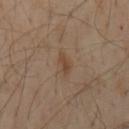Q: Was this lesion biopsied?
A: total-body-photography surveillance lesion; no biopsy
Q: Lesion size?
A: about 3 mm
Q: What did automated image analysis measure?
A: a lesion color around L≈44 a*≈16 b*≈29 in CIELAB, a lesion–skin lightness drop of about 7, and a normalized lesion–skin contrast near 6.5; a border-irregularity index near 4/10, a color-variation rating of about 0/10, and radial color variation of about 0; a classifier nevus-likeness of about 0/100 and a lesion-detection confidence of about 100/100
Q: How was the tile lit?
A: cross-polarized illumination
Q: Who is the patient?
A: male, aged 53 to 57
Q: What is the imaging modality?
A: total-body-photography crop, ~15 mm field of view
Q: Where on the body is the lesion?
A: the mid back